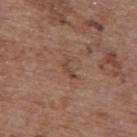<tbp_lesion>
<biopsy_status>not biopsied; imaged during a skin examination</biopsy_status>
<image>
  <source>total-body photography crop</source>
  <field_of_view_mm>15</field_of_view_mm>
</image>
<patient>
  <sex>female</sex>
  <age_approx>65</age_approx>
</patient>
<site>upper back</site>
<lighting>white-light</lighting>
<automated_metrics>
  <eccentricity>0.9</eccentricity>
  <shape_asymmetry>0.5</shape_asymmetry>
  <cielab_L>44</cielab_L>
  <cielab_a>20</cielab_a>
  <cielab_b>26</cielab_b>
  <vs_skin_darker_L>7.0</vs_skin_darker_L>
  <vs_skin_contrast_norm>6.0</vs_skin_contrast_norm>
  <nevus_likeness_0_100>0</nevus_likeness_0_100>
  <lesion_detection_confidence_0_100>100</lesion_detection_confidence_0_100>
</automated_metrics>
</tbp_lesion>